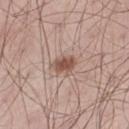The lesion is located on the left thigh. A male subject, approximately 55 years of age. A region of skin cropped from a whole-body photographic capture, roughly 15 mm wide. The recorded lesion diameter is about 3 mm. Imaged with white-light lighting.